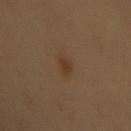Clinical summary: From the back. Measured at roughly 2.5 mm in maximum diameter. A lesion tile, about 15 mm wide, cut from a 3D total-body photograph. A female subject aged 58–62. The total-body-photography lesion software estimated a shape eccentricity near 0.75 and a symmetry-axis asymmetry near 0.2. The software also gave a lesion color around L≈36 a*≈16 b*≈29 in CIELAB, about 6 CIELAB-L* units darker than the surrounding skin, and a normalized lesion–skin contrast near 6.5.